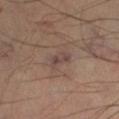Recorded during total-body skin imaging; not selected for excision or biopsy. An algorithmic analysis of the crop reported about 6 CIELAB-L* units darker than the surrounding skin and a normalized border contrast of about 6.5. It also reported a nevus-likeness score of about 0/100. A 15 mm crop from a total-body photograph taken for skin-cancer surveillance. From the left lower leg. Measured at roughly 2.5 mm in maximum diameter. A male subject aged around 45. This is a cross-polarized tile.